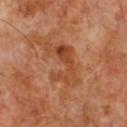* follow-up · catalogued during a skin exam; not biopsied
* acquisition · total-body-photography crop, ~15 mm field of view
* subject · male, roughly 60 years of age
* location · the chest
* diameter · ≈6 mm